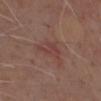follow-up = catalogued during a skin exam; not biopsied | imaging modality = 15 mm crop, total-body photography | location = the chest | patient = male, aged 68–72.